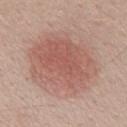Assessment:
Part of a total-body skin-imaging series; this lesion was reviewed on a skin check and was not flagged for biopsy.
Image and clinical context:
A male subject aged 38 to 42. Captured under white-light illumination. On the back. The total-body-photography lesion software estimated an average lesion color of about L≈58 a*≈22 b*≈25 (CIELAB) and roughly 9 lightness units darker than nearby skin. And it measured a border-irregularity index near 2/10, internal color variation of about 4.5 on a 0–10 scale, and a peripheral color-asymmetry measure near 1.5. It also reported a nevus-likeness score of about 100/100 and a detector confidence of about 100 out of 100 that the crop contains a lesion. A region of skin cropped from a whole-body photographic capture, roughly 15 mm wide.